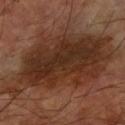<tbp_lesion>
<biopsy_status>not biopsied; imaged during a skin examination</biopsy_status>
<patient>
  <sex>male</sex>
  <age_approx>60</age_approx>
</patient>
<image>
  <source>total-body photography crop</source>
  <field_of_view_mm>15</field_of_view_mm>
</image>
<lighting>cross-polarized</lighting>
<site>right upper arm</site>
<lesion_size>
  <long_diameter_mm_approx>14.0</long_diameter_mm_approx>
</lesion_size>
</tbp_lesion>